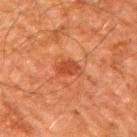follow-up = catalogued during a skin exam; not biopsied | patient = male, aged 58 to 62 | lesion size = ≈3 mm | location = the arm | image source = ~15 mm crop, total-body skin-cancer survey.